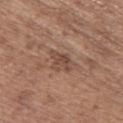Automated image analysis of the tile measured an area of roughly 5.5 mm² and a shape eccentricity near 0.75. The software also gave a border-irregularity index near 4/10 and internal color variation of about 2.5 on a 0–10 scale. The analysis additionally found a nevus-likeness score of about 0/100 and a lesion-detection confidence of about 100/100.
A 15 mm crop from a total-body photograph taken for skin-cancer surveillance.
A female patient aged 63–67.
The lesion is located on the upper back.